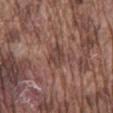Recorded during total-body skin imaging; not selected for excision or biopsy. A male subject, in their mid-70s. Located on the mid back. Imaged with white-light lighting. A 15 mm crop from a total-body photograph taken for skin-cancer surveillance. The lesion-visualizer software estimated a mean CIELAB color near L≈42 a*≈19 b*≈23 and a normalized lesion–skin contrast near 6.5. It also reported border irregularity of about 3 on a 0–10 scale, a color-variation rating of about 3/10, and radial color variation of about 1. And it measured a classifier nevus-likeness of about 0/100 and lesion-presence confidence of about 60/100. The recorded lesion diameter is about 3 mm.